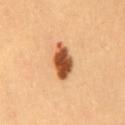Impression: No biopsy was performed on this lesion — it was imaged during a full skin examination and was not determined to be concerning. Clinical summary: A roughly 15 mm field-of-view crop from a total-body skin photograph. Longest diameter approximately 5 mm. The lesion-visualizer software estimated a footprint of about 8.5 mm², an outline eccentricity of about 0.85 (0 = round, 1 = elongated), and a shape-asymmetry score of about 0.2 (0 = symmetric). The analysis additionally found a mean CIELAB color near L≈53 a*≈28 b*≈40, roughly 22 lightness units darker than nearby skin, and a normalized lesion–skin contrast near 13.5. The tile uses cross-polarized illumination. A female subject aged 48 to 52.